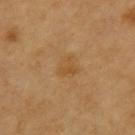Q: Was a biopsy performed?
A: catalogued during a skin exam; not biopsied
Q: What is the imaging modality?
A: total-body-photography crop, ~15 mm field of view
Q: Patient demographics?
A: male, about 60 years old
Q: Where on the body is the lesion?
A: the upper back